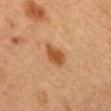Part of a total-body skin-imaging series; this lesion was reviewed on a skin check and was not flagged for biopsy. From the mid back. This is a cross-polarized tile. A close-up tile cropped from a whole-body skin photograph, about 15 mm across. A female patient, in their 60s. Longest diameter approximately 3.5 mm.